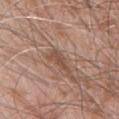Case summary:
• automated lesion analysis: an area of roughly 4 mm² and a shape eccentricity near 0.9; a border-irregularity index near 4/10, internal color variation of about 1.5 on a 0–10 scale, and peripheral color asymmetry of about 0.5; an automated nevus-likeness rating near 5 out of 100 and a lesion-detection confidence of about 100/100
• subject: male, in their 60s
• illumination: white-light
• lesion diameter: ~3 mm (longest diameter)
• image source: 15 mm crop, total-body photography
• anatomic site: the lower back